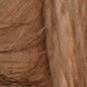The lesion was photographed on a routine skin check and not biopsied; there is no pathology result. The lesion's longest dimension is about 2 mm. Located on the upper back. A 15 mm crop from a total-body photograph taken for skin-cancer surveillance. A female subject in their 80s.